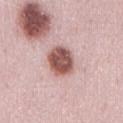| feature | finding |
|---|---|
| notes | catalogued during a skin exam; not biopsied |
| lighting | white-light illumination |
| subject | female, about 30 years old |
| location | the abdomen |
| automated lesion analysis | an average lesion color of about L≈54 a*≈23 b*≈23 (CIELAB), about 18 CIELAB-L* units darker than the surrounding skin, and a lesion-to-skin contrast of about 12 (normalized; higher = more distinct); a border-irregularity index near 1.5/10, internal color variation of about 4 on a 0–10 scale, and a peripheral color-asymmetry measure near 1; an automated nevus-likeness rating near 90 out of 100 and a lesion-detection confidence of about 100/100 |
| image | ~15 mm crop, total-body skin-cancer survey |
| size | ~4 mm (longest diameter) |